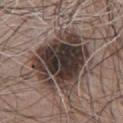The lesion was tiled from a total-body skin photograph and was not biopsied. Automated tile analysis of the lesion measured a lesion area of about 39 mm² and a symmetry-axis asymmetry near 0.25. It also reported a border-irregularity rating of about 4/10 and radial color variation of about 2.5. About 8 mm across. The lesion is on the front of the torso. This image is a 15 mm lesion crop taken from a total-body photograph. The patient is a male approximately 65 years of age. The tile uses white-light illumination.